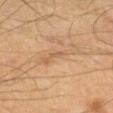- notes: total-body-photography surveillance lesion; no biopsy
- subject: male, aged approximately 55
- site: the left upper arm
- tile lighting: cross-polarized
- image source: ~15 mm tile from a whole-body skin photo